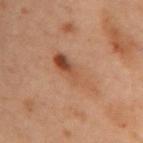notes: imaged on a skin check; not biopsied | patient: female, about 55 years old | lighting: cross-polarized | site: the left arm | imaging modality: ~15 mm crop, total-body skin-cancer survey | automated metrics: a footprint of about 10 mm², a shape eccentricity near 0.95, and a shape-asymmetry score of about 0.25 (0 = symmetric); a lesion–skin lightness drop of about 7 and a lesion-to-skin contrast of about 6.5 (normalized; higher = more distinct); a border-irregularity index near 4/10, internal color variation of about 9 on a 0–10 scale, and peripheral color asymmetry of about 3.5.